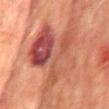Recorded during total-body skin imaging; not selected for excision or biopsy.
From the back.
Captured under cross-polarized illumination.
The lesion-visualizer software estimated a mean CIELAB color near L≈43 a*≈25 b*≈26, a lesion–skin lightness drop of about 10, and a normalized border contrast of about 8.5.
The subject is a male aged 73 to 77.
This image is a 15 mm lesion crop taken from a total-body photograph.
Approximately 12.5 mm at its widest.